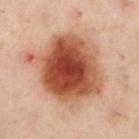Findings:
– notes — total-body-photography surveillance lesion; no biopsy
– patient — female, aged 58–62
– site — the right leg
– image — ~15 mm tile from a whole-body skin photo